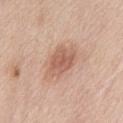biopsy status = no biopsy performed (imaged during a skin exam)
acquisition = 15 mm crop, total-body photography
location = the mid back
lesion diameter = about 4.5 mm
lighting = white-light
subject = female, approximately 60 years of age